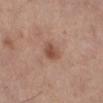The lesion was photographed on a routine skin check and not biopsied; there is no pathology result.
Cropped from a whole-body photographic skin survey; the tile spans about 15 mm.
From the right lower leg.
A female subject, aged approximately 65.